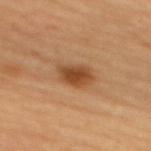The total-body-photography lesion software estimated a lesion color around L≈46 a*≈23 b*≈36 in CIELAB, a lesion–skin lightness drop of about 12, and a normalized border contrast of about 8.5. And it measured border irregularity of about 2 on a 0–10 scale, internal color variation of about 4.5 on a 0–10 scale, and radial color variation of about 1. And it measured a nevus-likeness score of about 95/100 and lesion-presence confidence of about 100/100. The lesion's longest dimension is about 3.5 mm. This is a cross-polarized tile. On the mid back. Cropped from a whole-body photographic skin survey; the tile spans about 15 mm. The patient is a female aged approximately 45.